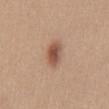biopsy_status: not biopsied; imaged during a skin examination
patient:
  sex: female
  age_approx: 30
image:
  source: total-body photography crop
  field_of_view_mm: 15
site: chest